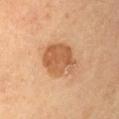Captured during whole-body skin photography for melanoma surveillance; the lesion was not biopsied. This is a cross-polarized tile. A region of skin cropped from a whole-body photographic capture, roughly 15 mm wide. A male patient aged approximately 55. On the arm. The lesion-visualizer software estimated a lesion color around L≈53 a*≈22 b*≈36 in CIELAB, about 11 CIELAB-L* units darker than the surrounding skin, and a normalized lesion–skin contrast near 8. And it measured a border-irregularity rating of about 2.5/10 and radial color variation of about 1. About 4.5 mm across.